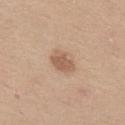Part of a total-body skin-imaging series; this lesion was reviewed on a skin check and was not flagged for biopsy.
The lesion-visualizer software estimated about 10 CIELAB-L* units darker than the surrounding skin and a lesion-to-skin contrast of about 7 (normalized; higher = more distinct). The software also gave a border-irregularity index near 2/10, internal color variation of about 2 on a 0–10 scale, and peripheral color asymmetry of about 0.5. It also reported lesion-presence confidence of about 100/100.
The subject is a female aged 38 to 42.
On the left thigh.
This image is a 15 mm lesion crop taken from a total-body photograph.
About 3.5 mm across.
Imaged with white-light lighting.